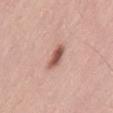{"biopsy_status": "not biopsied; imaged during a skin examination", "site": "lower back", "image": {"source": "total-body photography crop", "field_of_view_mm": 15}, "lesion_size": {"long_diameter_mm_approx": 3.0}, "patient": {"sex": "male", "age_approx": 50}}